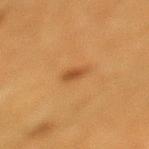follow-up: total-body-photography surveillance lesion; no biopsy | acquisition: ~15 mm tile from a whole-body skin photo | automated lesion analysis: a lesion color around L≈41 a*≈20 b*≈35 in CIELAB, roughly 8 lightness units darker than nearby skin, and a lesion-to-skin contrast of about 7 (normalized; higher = more distinct) | subject: female, aged 38–42 | lesion size: about 2.5 mm | tile lighting: cross-polarized | site: the left lower leg.